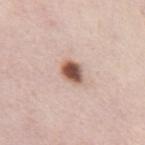Findings:
- biopsy status · total-body-photography surveillance lesion; no biopsy
- acquisition · 15 mm crop, total-body photography
- location · the front of the torso
- subject · female, aged 53 to 57
- illumination · white-light illumination
- size · ≈3 mm